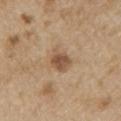Recorded during total-body skin imaging; not selected for excision or biopsy.
The subject is a male about 70 years old.
Located on the left upper arm.
Imaged with white-light lighting.
Measured at roughly 3 mm in maximum diameter.
This image is a 15 mm lesion crop taken from a total-body photograph.